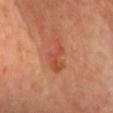{"biopsy_status": "not biopsied; imaged during a skin examination", "image": {"source": "total-body photography crop", "field_of_view_mm": 15}, "lighting": "cross-polarized", "patient": {"sex": "female", "age_approx": 40}, "automated_metrics": {"area_mm2_approx": 7.0, "shape_asymmetry": 0.5, "cielab_L": 52, "cielab_a": 30, "cielab_b": 37}, "site": "chest", "lesion_size": {"long_diameter_mm_approx": 4.5}}